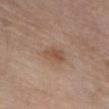The lesion was tiled from a total-body skin photograph and was not biopsied.
This image is a 15 mm lesion crop taken from a total-body photograph.
Automated image analysis of the tile measured a symmetry-axis asymmetry near 0.25.
This is a white-light tile.
A female patient aged 63 to 67.
Located on the chest.
Longest diameter approximately 3.5 mm.